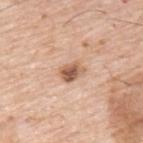Assessment: Part of a total-body skin-imaging series; this lesion was reviewed on a skin check and was not flagged for biopsy. Clinical summary: This is a white-light tile. Measured at roughly 3 mm in maximum diameter. A 15 mm close-up tile from a total-body photography series done for melanoma screening. Located on the back. The subject is a male roughly 70 years of age.